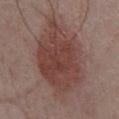notes = catalogued during a skin exam; not biopsied
acquisition = total-body-photography crop, ~15 mm field of view
location = the chest
illumination = white-light
subject = male, roughly 55 years of age
automated lesion analysis = a footprint of about 44 mm² and a shape eccentricity near 0.75; an average lesion color of about L≈42 a*≈20 b*≈22 (CIELAB), about 10 CIELAB-L* units darker than the surrounding skin, and a normalized border contrast of about 8; a border-irregularity index near 3/10, a color-variation rating of about 3.5/10, and a peripheral color-asymmetry measure near 1; a nevus-likeness score of about 90/100 and a lesion-detection confidence of about 100/100
size = ≈10 mm